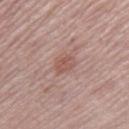Clinical impression:
Captured during whole-body skin photography for melanoma surveillance; the lesion was not biopsied.
Clinical summary:
The lesion's longest dimension is about 2.5 mm. A male subject, approximately 60 years of age. The tile uses white-light illumination. The lesion is on the right thigh. A lesion tile, about 15 mm wide, cut from a 3D total-body photograph.